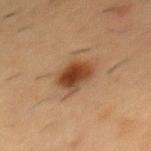The lesion was tiled from a total-body skin photograph and was not biopsied. Captured under cross-polarized illumination. Cropped from a whole-body photographic skin survey; the tile spans about 15 mm. Longest diameter approximately 3.5 mm. A male subject in their mid- to late 50s. From the chest.